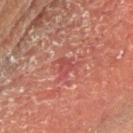Q: Was this lesion biopsied?
A: total-body-photography surveillance lesion; no biopsy